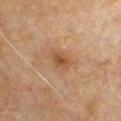{"biopsy_status": "not biopsied; imaged during a skin examination", "site": "chest", "patient": {"sex": "male", "age_approx": 65}, "image": {"source": "total-body photography crop", "field_of_view_mm": 15}, "lighting": "cross-polarized", "lesion_size": {"long_diameter_mm_approx": 2.5}}